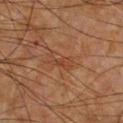• biopsy status · no biopsy performed (imaged during a skin exam)
• tile lighting · cross-polarized illumination
• subject · male, roughly 50 years of age
• image-analysis metrics · a symmetry-axis asymmetry near 0.3; an average lesion color of about L≈34 a*≈19 b*≈27 (CIELAB), a lesion–skin lightness drop of about 5, and a normalized lesion–skin contrast near 4.5; border irregularity of about 3.5 on a 0–10 scale; a classifier nevus-likeness of about 0/100 and a detector confidence of about 95 out of 100 that the crop contains a lesion
• imaging modality · ~15 mm crop, total-body skin-cancer survey
• location · the chest
• size · ~3.5 mm (longest diameter)